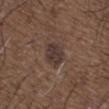biopsy status=catalogued during a skin exam; not biopsied | image-analysis metrics=a footprint of about 7.5 mm² and a shape eccentricity near 0.65 | tile lighting=white-light | image=total-body-photography crop, ~15 mm field of view | subject=male, aged approximately 50 | site=the upper back | lesion diameter=≈3.5 mm.